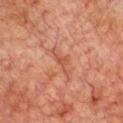Captured during whole-body skin photography for melanoma surveillance; the lesion was not biopsied. On the front of the torso. The subject is a male about 75 years old. The tile uses cross-polarized illumination. A 15 mm close-up tile from a total-body photography series done for melanoma screening. Longest diameter approximately 4 mm.